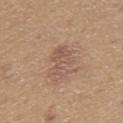follow-up — no biopsy performed (imaged during a skin exam)
illumination — white-light
acquisition — total-body-photography crop, ~15 mm field of view
subject — male, about 65 years old
site — the chest
automated lesion analysis — a lesion color around L≈55 a*≈18 b*≈27 in CIELAB, roughly 7 lightness units darker than nearby skin, and a normalized lesion–skin contrast near 5.5; an automated nevus-likeness rating near 0 out of 100 and lesion-presence confidence of about 100/100
size — ≈4.5 mm